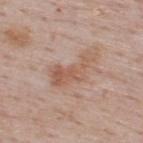automated lesion analysis = an eccentricity of roughly 0.9 and a symmetry-axis asymmetry near 0.55; border irregularity of about 6.5 on a 0–10 scale and peripheral color asymmetry of about 1; an automated nevus-likeness rating near 0 out of 100 and a detector confidence of about 100 out of 100 that the crop contains a lesion | illumination = white-light illumination | acquisition = total-body-photography crop, ~15 mm field of view | patient = male, in their 50s | location = the upper back | lesion diameter = ~6 mm (longest diameter).